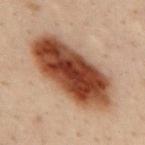notes: no biopsy performed (imaged during a skin exam); body site: the mid back; image: 15 mm crop, total-body photography; patient: male, approximately 30 years of age.